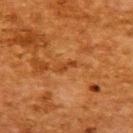Imaged during a routine full-body skin examination; the lesion was not biopsied and no histopathology is available. Cropped from a whole-body photographic skin survey; the tile spans about 15 mm. The lesion's longest dimension is about 2.5 mm. The patient is a female aged around 50. On the upper back.